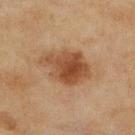{
  "lesion_size": {
    "long_diameter_mm_approx": 6.0
  },
  "patient": {
    "sex": "female",
    "age_approx": 60
  },
  "automated_metrics": {
    "area_mm2_approx": 16.0,
    "eccentricity": 0.75,
    "shape_asymmetry": 0.2,
    "border_irregularity_0_10": 3.0,
    "color_variation_0_10": 5.5
  },
  "lighting": "cross-polarized",
  "site": "right lower leg",
  "image": {
    "source": "total-body photography crop",
    "field_of_view_mm": 15
  }
}